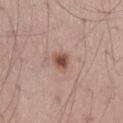Q: Was this lesion biopsied?
A: catalogued during a skin exam; not biopsied
Q: What is the imaging modality?
A: ~15 mm crop, total-body skin-cancer survey
Q: Where on the body is the lesion?
A: the leg
Q: Patient demographics?
A: male, aged approximately 55